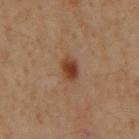– follow-up: no biopsy performed (imaged during a skin exam)